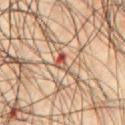Findings:
- biopsy status · catalogued during a skin exam; not biopsied
- location · the chest
- automated metrics · an area of roughly 3.5 mm², an eccentricity of roughly 0.85, and two-axis asymmetry of about 0.55; a classifier nevus-likeness of about 0/100 and lesion-presence confidence of about 100/100
- lesion diameter · about 3 mm
- lighting · cross-polarized
- patient · male, aged approximately 45
- imaging modality · ~15 mm tile from a whole-body skin photo